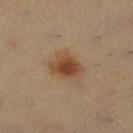Impression:
Recorded during total-body skin imaging; not selected for excision or biopsy.